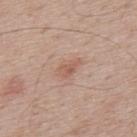Imaged during a routine full-body skin examination; the lesion was not biopsied and no histopathology is available.
A male patient approximately 70 years of age.
This is a white-light tile.
Cropped from a total-body skin-imaging series; the visible field is about 15 mm.
From the upper back.
The lesion's longest dimension is about 3 mm.